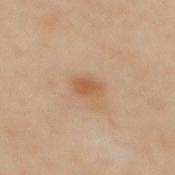Clinical impression: The lesion was photographed on a routine skin check and not biopsied; there is no pathology result. Background: The recorded lesion diameter is about 2.5 mm. The tile uses cross-polarized illumination. Cropped from a whole-body photographic skin survey; the tile spans about 15 mm. From the mid back. The patient is a female about 40 years old.